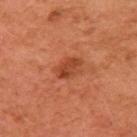Part of a total-body skin-imaging series; this lesion was reviewed on a skin check and was not flagged for biopsy. Located on the right upper arm. A female subject approximately 60 years of age. A 15 mm close-up extracted from a 3D total-body photography capture. Imaged with cross-polarized lighting. The lesion's longest dimension is about 3 mm.